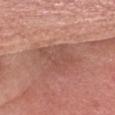follow-up: imaged on a skin check; not biopsied
body site: the head or neck
subject: male, in their mid-60s
acquisition: ~15 mm tile from a whole-body skin photo